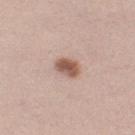No biopsy was performed on this lesion — it was imaged during a full skin examination and was not determined to be concerning. This is a white-light tile. A female subject about 40 years old. A lesion tile, about 15 mm wide, cut from a 3D total-body photograph. The lesion is on the left thigh.